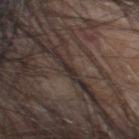Q: Was this lesion biopsied?
A: imaged on a skin check; not biopsied
Q: Where on the body is the lesion?
A: the head or neck
Q: What is the lesion's diameter?
A: ~2 mm (longest diameter)
Q: Patient demographics?
A: male, aged 53–57
Q: Automated lesion metrics?
A: a shape-asymmetry score of about 0.3 (0 = symmetric); a nevus-likeness score of about 0/100 and lesion-presence confidence of about 0/100
Q: Illumination type?
A: white-light illumination
Q: What is the imaging modality?
A: total-body-photography crop, ~15 mm field of view